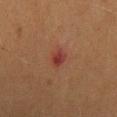Assessment: No biopsy was performed on this lesion — it was imaged during a full skin examination and was not determined to be concerning. Context: The lesion is located on the head or neck. A female subject, about 60 years old. Captured under cross-polarized illumination. A lesion tile, about 15 mm wide, cut from a 3D total-body photograph.